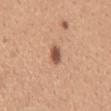Recorded during total-body skin imaging; not selected for excision or biopsy. The lesion-visualizer software estimated a footprint of about 4 mm², an eccentricity of roughly 0.8, and a shape-asymmetry score of about 0.2 (0 = symmetric). The analysis additionally found a border-irregularity rating of about 2/10, internal color variation of about 3.5 on a 0–10 scale, and radial color variation of about 1. The software also gave a classifier nevus-likeness of about 95/100 and lesion-presence confidence of about 100/100. Imaged with white-light lighting. A female subject, in their 30s. On the back. Longest diameter approximately 3 mm. A 15 mm close-up extracted from a 3D total-body photography capture.